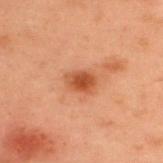follow-up = no biopsy performed (imaged during a skin exam); diameter = about 3 mm; subject = male, aged approximately 55; image = ~15 mm tile from a whole-body skin photo; site = the upper back; lighting = cross-polarized illumination.